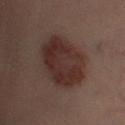notes: catalogued during a skin exam; not biopsied
size: ~6.5 mm (longest diameter)
subject: male, aged around 55
acquisition: ~15 mm tile from a whole-body skin photo
illumination: cross-polarized illumination
body site: the left lower leg